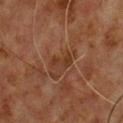No biopsy was performed on this lesion — it was imaged during a full skin examination and was not determined to be concerning. Cropped from a total-body skin-imaging series; the visible field is about 15 mm. Located on the front of the torso. Longest diameter approximately 3 mm. The patient is a male aged 58 to 62. Imaged with cross-polarized lighting. The lesion-visualizer software estimated an area of roughly 4 mm², an outline eccentricity of about 0.85 (0 = round, 1 = elongated), and a shape-asymmetry score of about 0.35 (0 = symmetric). And it measured border irregularity of about 4 on a 0–10 scale and peripheral color asymmetry of about 0.5. And it measured an automated nevus-likeness rating near 0 out of 100 and a detector confidence of about 100 out of 100 that the crop contains a lesion.